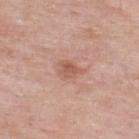<tbp_lesion>
<site>upper back</site>
<lighting>white-light</lighting>
<patient>
  <sex>male</sex>
  <age_approx>55</age_approx>
</patient>
<image>
  <source>total-body photography crop</source>
  <field_of_view_mm>15</field_of_view_mm>
</image>
<automated_metrics>
  <area_mm2_approx>3.5</area_mm2_approx>
  <shape_asymmetry>0.4</shape_asymmetry>
  <cielab_L>56</cielab_L>
  <cielab_a>24</cielab_a>
  <cielab_b>29</cielab_b>
  <vs_skin_darker_L>9.0</vs_skin_darker_L>
  <vs_skin_contrast_norm>6.5</vs_skin_contrast_norm>
  <nevus_likeness_0_100>35</nevus_likeness_0_100>
  <lesion_detection_confidence_0_100>100</lesion_detection_confidence_0_100>
</automated_metrics>
<lesion_size>
  <long_diameter_mm_approx>2.5</long_diameter_mm_approx>
</lesion_size>
</tbp_lesion>